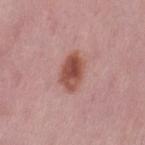| key | value |
|---|---|
| image | total-body-photography crop, ~15 mm field of view |
| location | the left thigh |
| patient | female, aged 48 to 52 |
| diameter | about 4.5 mm |
| lighting | white-light |